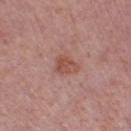Impression:
The lesion was tiled from a total-body skin photograph and was not biopsied.
Context:
A female subject about 50 years old. Measured at roughly 3 mm in maximum diameter. The lesion is on the leg. Captured under white-light illumination. A 15 mm close-up extracted from a 3D total-body photography capture.